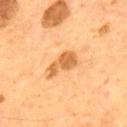Notes:
– patient — male, approximately 55 years of age
– diameter — ≈4.5 mm
– site — the upper back
– image source — ~15 mm crop, total-body skin-cancer survey
– illumination — cross-polarized illumination